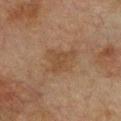Clinical impression: The lesion was photographed on a routine skin check and not biopsied; there is no pathology result. Image and clinical context: The subject is a male roughly 75 years of age. The lesion-visualizer software estimated an average lesion color of about L≈38 a*≈15 b*≈27 (CIELAB), about 5 CIELAB-L* units darker than the surrounding skin, and a normalized border contrast of about 5.5. The software also gave a border-irregularity index near 4.5/10 and peripheral color asymmetry of about 0.5. And it measured an automated nevus-likeness rating near 0 out of 100 and lesion-presence confidence of about 100/100. Measured at roughly 4 mm in maximum diameter. On the chest. Cropped from a total-body skin-imaging series; the visible field is about 15 mm. This is a cross-polarized tile.